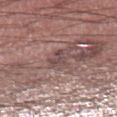Clinical impression: Imaged during a routine full-body skin examination; the lesion was not biopsied and no histopathology is available. Context: The tile uses white-light illumination. A 15 mm close-up extracted from a 3D total-body photography capture. About 2.5 mm across. From the right lower leg. The patient is a male in their mid-40s.